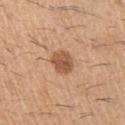The lesion was photographed on a routine skin check and not biopsied; there is no pathology result.
A roughly 15 mm field-of-view crop from a total-body skin photograph.
Longest diameter approximately 3 mm.
The lesion is on the right upper arm.
The patient is a male aged around 60.